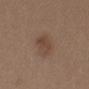notes: no biopsy performed (imaged during a skin exam) | patient: female, aged approximately 40 | size: about 3.5 mm | lighting: white-light | location: the abdomen | acquisition: ~15 mm tile from a whole-body skin photo | automated metrics: a footprint of about 6 mm², an outline eccentricity of about 0.75 (0 = round, 1 = elongated), and a symmetry-axis asymmetry near 0.25; border irregularity of about 2.5 on a 0–10 scale, a within-lesion color-variation index near 3/10, and radial color variation of about 1; an automated nevus-likeness rating near 30 out of 100 and a lesion-detection confidence of about 100/100.